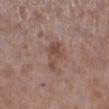Part of a total-body skin-imaging series; this lesion was reviewed on a skin check and was not flagged for biopsy.
The lesion's longest dimension is about 4 mm.
A 15 mm close-up extracted from a 3D total-body photography capture.
From the leg.
The patient is a male about 70 years old.
Automated tile analysis of the lesion measured an area of roughly 6.5 mm², a shape eccentricity near 0.85, and a shape-asymmetry score of about 0.4 (0 = symmetric).